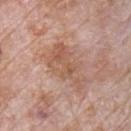<tbp_lesion>
  <biopsy_status>not biopsied; imaged during a skin examination</biopsy_status>
  <lesion_size>
    <long_diameter_mm_approx>6.0</long_diameter_mm_approx>
  </lesion_size>
  <automated_metrics>
    <cielab_L>56</cielab_L>
    <cielab_a>21</cielab_a>
    <cielab_b>30</cielab_b>
    <vs_skin_contrast_norm>6.5</vs_skin_contrast_norm>
    <nevus_likeness_0_100>0</nevus_likeness_0_100>
  </automated_metrics>
  <patient>
    <sex>male</sex>
    <age_approx>70</age_approx>
  </patient>
  <lighting>white-light</lighting>
  <image>
    <source>total-body photography crop</source>
    <field_of_view_mm>15</field_of_view_mm>
  </image>
  <site>chest</site>
</tbp_lesion>